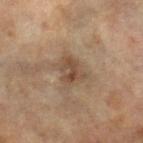Impression: Recorded during total-body skin imaging; not selected for excision or biopsy. Clinical summary: The patient is a female in their mid- to late 60s. The lesion is located on the left leg. The recorded lesion diameter is about 3.5 mm. A lesion tile, about 15 mm wide, cut from a 3D total-body photograph. The tile uses cross-polarized illumination.